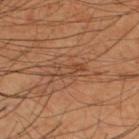  lighting: cross-polarized
  site: upper back
  lesion_size:
    long_diameter_mm_approx: 3.5
  image:
    source: total-body photography crop
    field_of_view_mm: 15
  patient:
    sex: male
    age_approx: 50